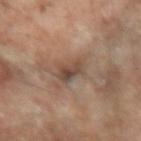Imaged during a routine full-body skin examination; the lesion was not biopsied and no histopathology is available. The lesion is on the left forearm. A female subject, aged 68 to 72. A 15 mm crop from a total-body photograph taken for skin-cancer surveillance.